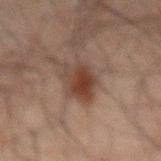workup=no biopsy performed (imaged during a skin exam) | patient=male, aged around 60 | acquisition=15 mm crop, total-body photography | site=the abdomen.